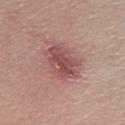No biopsy was performed on this lesion — it was imaged during a full skin examination and was not determined to be concerning. Automated tile analysis of the lesion measured a border-irregularity index near 2/10, a within-lesion color-variation index near 6.5/10, and radial color variation of about 2.5. The software also gave a nevus-likeness score of about 10/100 and a lesion-detection confidence of about 100/100. Longest diameter approximately 5 mm. On the left lower leg. This is a white-light tile. Cropped from a whole-body photographic skin survey; the tile spans about 15 mm. A male patient, approximately 45 years of age.